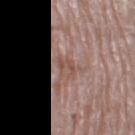* notes · total-body-photography surveillance lesion; no biopsy
* image source · ~15 mm tile from a whole-body skin photo
* subject · female, aged 73 to 77
* location · the left thigh
* illumination · white-light illumination
* lesion size · ~3.5 mm (longest diameter)